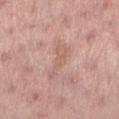| field | value |
|---|---|
| notes | no biopsy performed (imaged during a skin exam) |
| subject | female, aged 23 to 27 |
| lighting | white-light |
| image | ~15 mm tile from a whole-body skin photo |
| site | the leg |
| diameter | ≈4.5 mm |